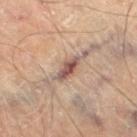Clinical impression:
Part of a total-body skin-imaging series; this lesion was reviewed on a skin check and was not flagged for biopsy.
Image and clinical context:
A roughly 15 mm field-of-view crop from a total-body skin photograph. On the right thigh. The patient is a male aged around 70. About 3.5 mm across.